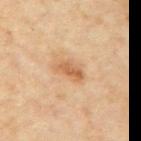Context: A female patient aged 58 to 62. Located on the right upper arm. Captured under cross-polarized illumination. A 15 mm close-up extracted from a 3D total-body photography capture.